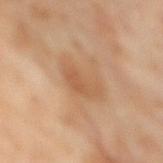workup = no biopsy performed (imaged during a skin exam) | patient = male, roughly 60 years of age | acquisition = ~15 mm crop, total-body skin-cancer survey | body site = the mid back.